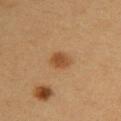Findings:
• notes: catalogued during a skin exam; not biopsied
• body site: the upper back
• automated metrics: a normalized lesion–skin contrast near 7.5; border irregularity of about 1 on a 0–10 scale, internal color variation of about 2 on a 0–10 scale, and a peripheral color-asymmetry measure near 0.5
• subject: female, in their 30s
• lesion diameter: ≈2.5 mm
• acquisition: 15 mm crop, total-body photography